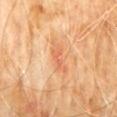workup — imaged on a skin check; not biopsied
body site — the abdomen
patient — male, aged 58 to 62
image source — ~15 mm crop, total-body skin-cancer survey